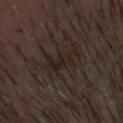The lesion was photographed on a routine skin check and not biopsied; there is no pathology result. The lesion is located on the head or neck. The tile uses cross-polarized illumination. The subject is a male aged around 55. A 15 mm crop from a total-body photograph taken for skin-cancer surveillance. Longest diameter approximately 5.5 mm.